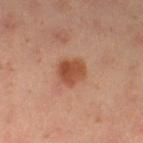Clinical impression:
Captured during whole-body skin photography for melanoma surveillance; the lesion was not biopsied.
Acquisition and patient details:
Automated tile analysis of the lesion measured a footprint of about 8 mm² and a symmetry-axis asymmetry near 0.2. The analysis additionally found border irregularity of about 2 on a 0–10 scale and radial color variation of about 1.5. A lesion tile, about 15 mm wide, cut from a 3D total-body photograph. The subject is a female approximately 40 years of age. The lesion's longest dimension is about 3.5 mm. Located on the right lower leg. Imaged with cross-polarized lighting.